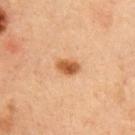<record>
<biopsy_status>not biopsied; imaged during a skin examination</biopsy_status>
<image>
  <source>total-body photography crop</source>
  <field_of_view_mm>15</field_of_view_mm>
</image>
<lesion_size>
  <long_diameter_mm_approx>2.5</long_diameter_mm_approx>
</lesion_size>
<lighting>cross-polarized</lighting>
<site>right upper arm</site>
<patient>
  <sex>male</sex>
  <age_approx>60</age_approx>
</patient>
</record>